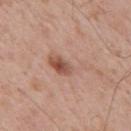Notes:
* follow-up: catalogued during a skin exam; not biopsied
* size: ≈5.5 mm
* anatomic site: the mid back
* lighting: white-light
* imaging modality: 15 mm crop, total-body photography
* patient: male, aged 63–67
* image-analysis metrics: a lesion area of about 9 mm²; an average lesion color of about L≈55 a*≈21 b*≈28 (CIELAB) and a lesion–skin lightness drop of about 9; a border-irregularity rating of about 6.5/10, a color-variation rating of about 7/10, and a peripheral color-asymmetry measure near 2.5